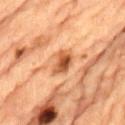Q: Who is the patient?
A: male, aged 83 or over
Q: Illumination type?
A: cross-polarized
Q: How large is the lesion?
A: ~3.5 mm (longest diameter)
Q: How was this image acquired?
A: ~15 mm tile from a whole-body skin photo
Q: What is the anatomic site?
A: the back